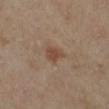| field | value |
|---|---|
| patient | female, about 70 years old |
| image | ~15 mm tile from a whole-body skin photo |
| location | the leg |